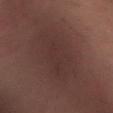Clinical impression: The lesion was tiled from a total-body skin photograph and was not biopsied. Image and clinical context: The lesion is on the left forearm. A 15 mm close-up tile from a total-body photography series done for melanoma screening. Automated image analysis of the tile measured a lesion color around L≈24 a*≈14 b*≈16 in CIELAB, about 4 CIELAB-L* units darker than the surrounding skin, and a normalized lesion–skin contrast near 4.5. The software also gave a nevus-likeness score of about 0/100. A male subject, roughly 30 years of age. About 7 mm across. Imaged with cross-polarized lighting.